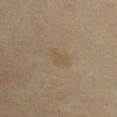| feature | finding |
|---|---|
| biopsy status | total-body-photography surveillance lesion; no biopsy |
| imaging modality | ~15 mm crop, total-body skin-cancer survey |
| patient | male, about 45 years old |
| body site | the chest |
| tile lighting | cross-polarized |